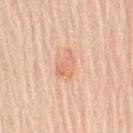Longest diameter approximately 3.5 mm.
Located on the right upper arm.
A female subject, aged 68 to 72.
A roughly 15 mm field-of-view crop from a total-body skin photograph.
This is a white-light tile.